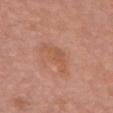A female subject, about 60 years old. The tile uses white-light illumination. A roughly 15 mm field-of-view crop from a total-body skin photograph. The lesion's longest dimension is about 4.5 mm. On the chest.